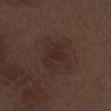Q: Was this lesion biopsied?
A: imaged on a skin check; not biopsied
Q: How was the tile lit?
A: white-light illumination
Q: How was this image acquired?
A: 15 mm crop, total-body photography
Q: Automated lesion metrics?
A: an eccentricity of roughly 0.6 and two-axis asymmetry of about 0.4; a classifier nevus-likeness of about 0/100 and a lesion-detection confidence of about 100/100
Q: What is the lesion's diameter?
A: about 4 mm
Q: What are the patient's age and sex?
A: male, aged approximately 70
Q: Lesion location?
A: the right thigh